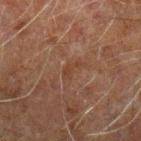Clinical impression: Captured during whole-body skin photography for melanoma surveillance; the lesion was not biopsied. Context: A region of skin cropped from a whole-body photographic capture, roughly 15 mm wide. The total-body-photography lesion software estimated a footprint of about 2 mm², a shape eccentricity near 0.9, and two-axis asymmetry of about 0.5. And it measured a border-irregularity index near 5.5/10, internal color variation of about 0 on a 0–10 scale, and radial color variation of about 0. It also reported a detector confidence of about 100 out of 100 that the crop contains a lesion. Measured at roughly 2.5 mm in maximum diameter. Imaged with cross-polarized lighting. The patient is a male aged approximately 60. On the left forearm.